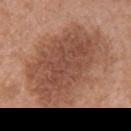follow-up: total-body-photography surveillance lesion; no biopsy | acquisition: ~15 mm tile from a whole-body skin photo | size: about 11.5 mm | body site: the right upper arm | patient: female, roughly 40 years of age.